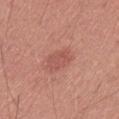• notes: total-body-photography surveillance lesion; no biopsy
• image source: total-body-photography crop, ~15 mm field of view
• subject: male, aged 63 to 67
• body site: the left thigh